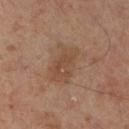{"biopsy_status": "not biopsied; imaged during a skin examination", "automated_metrics": {"area_mm2_approx": 11.0, "eccentricity": 0.85, "shape_asymmetry": 0.25, "cielab_L": 46, "cielab_a": 18, "cielab_b": 29, "vs_skin_darker_L": 7.0, "vs_skin_contrast_norm": 6.0, "border_irregularity_0_10": 4.0, "color_variation_0_10": 3.0, "peripheral_color_asymmetry": 1.0}, "site": "left leg", "image": {"source": "total-body photography crop", "field_of_view_mm": 15}, "lesion_size": {"long_diameter_mm_approx": 5.0}, "patient": {"sex": "male", "age_approx": 50}}